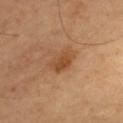Q: Was this lesion biopsied?
A: imaged on a skin check; not biopsied
Q: What kind of image is this?
A: ~15 mm crop, total-body skin-cancer survey
Q: Lesion location?
A: the chest
Q: What are the patient's age and sex?
A: male, aged approximately 50
Q: Lesion size?
A: about 3.5 mm
Q: What lighting was used for the tile?
A: cross-polarized